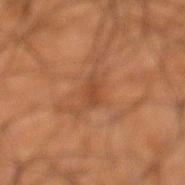<record>
  <biopsy_status>not biopsied; imaged during a skin examination</biopsy_status>
  <patient>
    <sex>male</sex>
    <age_approx>55</age_approx>
  </patient>
  <lighting>cross-polarized</lighting>
  <automated_metrics>
    <area_mm2_approx>3.5</area_mm2_approx>
    <eccentricity>0.9</eccentricity>
    <shape_asymmetry>0.3</shape_asymmetry>
    <vs_skin_darker_L>7.0</vs_skin_darker_L>
    <vs_skin_contrast_norm>5.5</vs_skin_contrast_norm>
    <nevus_likeness_0_100>0</nevus_likeness_0_100>
    <lesion_detection_confidence_0_100>95</lesion_detection_confidence_0_100>
  </automated_metrics>
  <lesion_size>
    <long_diameter_mm_approx>3.0</long_diameter_mm_approx>
  </lesion_size>
  <image>
    <source>total-body photography crop</source>
    <field_of_view_mm>15</field_of_view_mm>
  </image>
  <site>left lower leg</site>
</record>